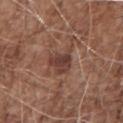workup: total-body-photography surveillance lesion; no biopsy
subject: male, in their 70s
tile lighting: white-light
location: the right upper arm
image source: 15 mm crop, total-body photography
image-analysis metrics: a footprint of about 7.5 mm², an outline eccentricity of about 0.65 (0 = round, 1 = elongated), and a shape-asymmetry score of about 0.35 (0 = symmetric)
lesion size: ≈4 mm